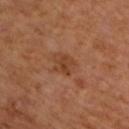Impression: The lesion was tiled from a total-body skin photograph and was not biopsied. Acquisition and patient details: Cropped from a whole-body photographic skin survey; the tile spans about 15 mm. Captured under cross-polarized illumination. About 3.5 mm across. A male subject in their mid-60s.